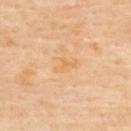| key | value |
|---|---|
| biopsy status | imaged on a skin check; not biopsied |